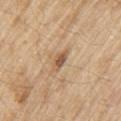Captured during whole-body skin photography for melanoma surveillance; the lesion was not biopsied.
Located on the left upper arm.
Captured under white-light illumination.
Automated image analysis of the tile measured a mean CIELAB color near L≈56 a*≈18 b*≈34, about 11 CIELAB-L* units darker than the surrounding skin, and a normalized border contrast of about 7.5. It also reported border irregularity of about 3 on a 0–10 scale and a color-variation rating of about 3/10. The software also gave an automated nevus-likeness rating near 0 out of 100.
This image is a 15 mm lesion crop taken from a total-body photograph.
Longest diameter approximately 3 mm.
The patient is a male approximately 70 years of age.